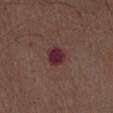follow-up: no biopsy performed (imaged during a skin exam) | patient: male, about 70 years old | body site: the abdomen | acquisition: ~15 mm tile from a whole-body skin photo | image-analysis metrics: a lesion area of about 5 mm², an outline eccentricity of about 0.5 (0 = round, 1 = elongated), and two-axis asymmetry of about 0.2; an average lesion color of about L≈29 a*≈23 b*≈16 (CIELAB) and a normalized lesion–skin contrast near 12; a classifier nevus-likeness of about 0/100 and a detector confidence of about 100 out of 100 that the crop contains a lesion.